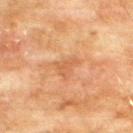Recorded during total-body skin imaging; not selected for excision or biopsy. The subject is a male approximately 75 years of age. The tile uses cross-polarized illumination. The lesion-visualizer software estimated a footprint of about 4.5 mm². The analysis additionally found a mean CIELAB color near L≈50 a*≈21 b*≈34, about 6 CIELAB-L* units darker than the surrounding skin, and a normalized lesion–skin contrast near 4.5. The analysis additionally found a border-irregularity rating of about 5/10 and peripheral color asymmetry of about 0.5. Measured at roughly 3 mm in maximum diameter. On the upper back. This image is a 15 mm lesion crop taken from a total-body photograph.